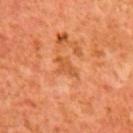  biopsy_status: not biopsied; imaged during a skin examination
  image:
    source: total-body photography crop
    field_of_view_mm: 15
  site: upper back
  patient:
    sex: male
    age_approx: 65
  automated_metrics:
    nevus_likeness_0_100: 0
    lesion_detection_confidence_0_100: 100
  lesion_size:
    long_diameter_mm_approx: 3.0